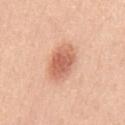Q: Patient demographics?
A: male, in their 50s
Q: What is the anatomic site?
A: the chest
Q: What is the lesion's diameter?
A: about 5 mm
Q: What did automated image analysis measure?
A: an area of roughly 11 mm², a shape eccentricity near 0.8, and a symmetry-axis asymmetry near 0.15
Q: What kind of image is this?
A: ~15 mm tile from a whole-body skin photo